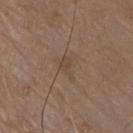Clinical impression: Captured during whole-body skin photography for melanoma surveillance; the lesion was not biopsied. Image and clinical context: A 15 mm close-up extracted from a 3D total-body photography capture. The lesion-visualizer software estimated a classifier nevus-likeness of about 0/100 and lesion-presence confidence of about 100/100. Located on the chest. Approximately 2.5 mm at its widest. Captured under white-light illumination. A male patient, in their 80s.